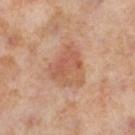Q: Is there a histopathology result?
A: total-body-photography surveillance lesion; no biopsy
Q: Lesion size?
A: about 4.5 mm
Q: What did automated image analysis measure?
A: radial color variation of about 1.5
Q: How was the tile lit?
A: cross-polarized illumination
Q: Patient demographics?
A: female, in their mid- to late 50s
Q: Lesion location?
A: the leg
Q: What kind of image is this?
A: 15 mm crop, total-body photography